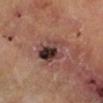Part of a total-body skin-imaging series; this lesion was reviewed on a skin check and was not flagged for biopsy. The subject is a male aged 63 to 67. From the left leg. The tile uses cross-polarized illumination. A roughly 15 mm field-of-view crop from a total-body skin photograph. The lesion-visualizer software estimated an eccentricity of roughly 0.5 and a symmetry-axis asymmetry near 0.25. Measured at roughly 4.5 mm in maximum diameter.